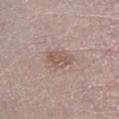A male patient, aged around 50. Located on the left lower leg. A region of skin cropped from a whole-body photographic capture, roughly 15 mm wide. The tile uses white-light illumination. Automated image analysis of the tile measured a lesion area of about 6.5 mm² and a shape eccentricity near 0.7. And it measured a border-irregularity index near 2.5/10, a color-variation rating of about 2.5/10, and peripheral color asymmetry of about 1. The analysis additionally found a classifier nevus-likeness of about 5/100 and lesion-presence confidence of about 100/100.